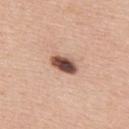  biopsy_status: not biopsied; imaged during a skin examination
  image:
    source: total-body photography crop
    field_of_view_mm: 15
  lesion_size:
    long_diameter_mm_approx: 3.5
  site: upper back
  lighting: white-light
  patient:
    sex: male
    age_approx: 60